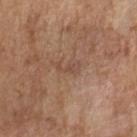follow-up=total-body-photography surveillance lesion; no biopsy | location=the left forearm | patient=female, about 70 years old | tile lighting=cross-polarized illumination | lesion diameter=~3 mm (longest diameter) | imaging modality=~15 mm tile from a whole-body skin photo.